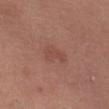Notes:
– workup — total-body-photography surveillance lesion; no biopsy
– automated metrics — a footprint of about 5 mm², a shape eccentricity near 0.85, and a symmetry-axis asymmetry near 0.35
– acquisition — 15 mm crop, total-body photography
– patient — female, aged around 65
– illumination — white-light
– anatomic site — the abdomen
– lesion size — about 3.5 mm